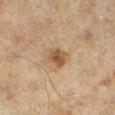Recorded during total-body skin imaging; not selected for excision or biopsy. The recorded lesion diameter is about 3 mm. A female patient, approximately 60 years of age. A lesion tile, about 15 mm wide, cut from a 3D total-body photograph. Located on the leg. Captured under cross-polarized illumination.